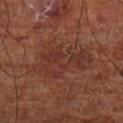Impression:
Recorded during total-body skin imaging; not selected for excision or biopsy.
Clinical summary:
Measured at roughly 6.5 mm in maximum diameter. Located on the left lower leg. This is a cross-polarized tile. A 15 mm close-up tile from a total-body photography series done for melanoma screening. The subject is a male aged 68 to 72.